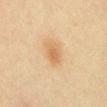The lesion was photographed on a routine skin check and not biopsied; there is no pathology result.
Longest diameter approximately 3.5 mm.
A female subject, aged around 45.
The tile uses cross-polarized illumination.
Located on the back.
A roughly 15 mm field-of-view crop from a total-body skin photograph.